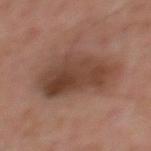Assessment: Imaged during a routine full-body skin examination; the lesion was not biopsied and no histopathology is available. Background: Approximately 9 mm at its widest. The lesion is located on the upper back. Captured under cross-polarized illumination. A 15 mm crop from a total-body photograph taken for skin-cancer surveillance. A male subject roughly 50 years of age.